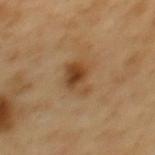{"biopsy_status": "not biopsied; imaged during a skin examination", "lesion_size": {"long_diameter_mm_approx": 4.0}, "site": "mid back", "image": {"source": "total-body photography crop", "field_of_view_mm": 15}, "patient": {"sex": "male", "age_approx": 50}, "lighting": "cross-polarized"}